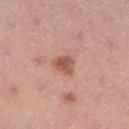Impression:
No biopsy was performed on this lesion — it was imaged during a full skin examination and was not determined to be concerning.
Context:
On the right lower leg. A female patient about 35 years old. About 3 mm across. Cropped from a total-body skin-imaging series; the visible field is about 15 mm. The tile uses white-light illumination.